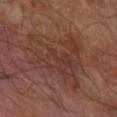<record>
<biopsy_status>not biopsied; imaged during a skin examination</biopsy_status>
<lighting>cross-polarized</lighting>
<patient>
  <sex>male</sex>
  <age_approx>70</age_approx>
</patient>
<site>right forearm</site>
<lesion_size>
  <long_diameter_mm_approx>8.5</long_diameter_mm_approx>
</lesion_size>
<image>
  <source>total-body photography crop</source>
  <field_of_view_mm>15</field_of_view_mm>
</image>
</record>